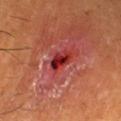Impression:
No biopsy was performed on this lesion — it was imaged during a full skin examination and was not determined to be concerning.
Background:
A lesion tile, about 15 mm wide, cut from a 3D total-body photograph. From the right lower leg. A male patient aged approximately 60. Longest diameter approximately 3 mm. Captured under cross-polarized illumination.